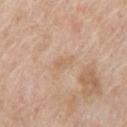The patient is a male about 65 years old.
A roughly 15 mm field-of-view crop from a total-body skin photograph.
On the chest.
Automated image analysis of the tile measured a footprint of about 3 mm², a shape eccentricity near 0.85, and two-axis asymmetry of about 0.35. And it measured roughly 6 lightness units darker than nearby skin.
Approximately 3 mm at its widest.